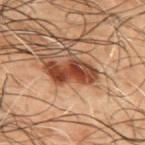<tbp_lesion>
<biopsy_status>not biopsied; imaged during a skin examination</biopsy_status>
<image>
  <source>total-body photography crop</source>
  <field_of_view_mm>15</field_of_view_mm>
</image>
<patient>
  <sex>male</sex>
  <age_approx>50</age_approx>
</patient>
<automated_metrics>
  <cielab_L>32</cielab_L>
  <cielab_a>21</cielab_a>
  <cielab_b>26</cielab_b>
  <vs_skin_darker_L>14.0</vs_skin_darker_L>
  <border_irregularity_0_10>4.5</border_irregularity_0_10>
  <color_variation_0_10>5.5</color_variation_0_10>
  <peripheral_color_asymmetry>1.5</peripheral_color_asymmetry>
  <nevus_likeness_0_100>85</nevus_likeness_0_100>
  <lesion_detection_confidence_0_100>100</lesion_detection_confidence_0_100>
</automated_metrics>
<site>upper back</site>
<lighting>cross-polarized</lighting>
</tbp_lesion>